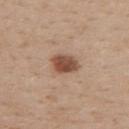Imaged during a routine full-body skin examination; the lesion was not biopsied and no histopathology is available. A 15 mm crop from a total-body photograph taken for skin-cancer surveillance. The lesion is located on the upper back. Approximately 3.5 mm at its widest. The patient is a male about 70 years old. Imaged with white-light lighting.